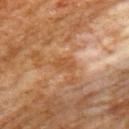  biopsy_status: not biopsied; imaged during a skin examination
  lesion_size:
    long_diameter_mm_approx: 2.5
  automated_metrics:
    cielab_L: 51
    cielab_a: 24
    cielab_b: 39
    vs_skin_darker_L: 7.0
    vs_skin_contrast_norm: 6.0
    border_irregularity_0_10: 2.5
    color_variation_0_10: 1.0
    peripheral_color_asymmetry: 0.5
  patient:
    sex: female
    age_approx: 50
  image:
    source: total-body photography crop
    field_of_view_mm: 15
  lighting: cross-polarized
  site: arm